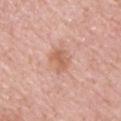biopsy status: no biopsy performed (imaged during a skin exam)
TBP lesion metrics: a lesion area of about 5.5 mm², an outline eccentricity of about 0.75 (0 = round, 1 = elongated), and a symmetry-axis asymmetry near 0.3; an average lesion color of about L≈61 a*≈23 b*≈31 (CIELAB), about 9 CIELAB-L* units darker than the surrounding skin, and a lesion-to-skin contrast of about 6.5 (normalized; higher = more distinct); a border-irregularity rating of about 3/10, a within-lesion color-variation index near 3/10, and a peripheral color-asymmetry measure near 1
imaging modality: ~15 mm tile from a whole-body skin photo
illumination: white-light
subject: male, about 50 years old
site: the chest
lesion diameter: about 3 mm